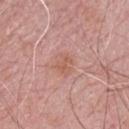Part of a total-body skin-imaging series; this lesion was reviewed on a skin check and was not flagged for biopsy. An algorithmic analysis of the crop reported a mean CIELAB color near L≈58 a*≈24 b*≈28, about 6 CIELAB-L* units darker than the surrounding skin, and a normalized lesion–skin contrast near 5.5. The software also gave a border-irregularity index near 3.5/10 and a within-lesion color-variation index near 1.5/10. It also reported a nevus-likeness score of about 5/100 and a detector confidence of about 100 out of 100 that the crop contains a lesion. This is a white-light tile. Longest diameter approximately 2.5 mm. A male subject, aged approximately 65. The lesion is on the chest. A 15 mm close-up tile from a total-body photography series done for melanoma screening.